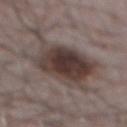Image and clinical context: Located on the abdomen. This is a white-light tile. A 15 mm crop from a total-body photograph taken for skin-cancer surveillance. The recorded lesion diameter is about 11.5 mm. A male patient in their mid-70s.